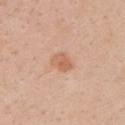| field | value |
|---|---|
| follow-up | total-body-photography surveillance lesion; no biopsy |
| subject | male, in their 30s |
| image source | 15 mm crop, total-body photography |
| anatomic site | the arm |